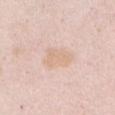* anatomic site · the abdomen
* image-analysis metrics · an area of roughly 7.5 mm², an outline eccentricity of about 0.8 (0 = round, 1 = elongated), and a shape-asymmetry score of about 0.25 (0 = symmetric); a border-irregularity rating of about 3/10, internal color variation of about 1.5 on a 0–10 scale, and peripheral color asymmetry of about 0.5; a nevus-likeness score of about 0/100
* illumination · white-light illumination
* lesion size · ≈4 mm
* subject · female, in their 40s
* imaging modality · ~15 mm crop, total-body skin-cancer survey